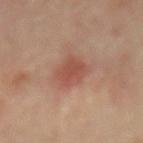Imaged during a routine full-body skin examination; the lesion was not biopsied and no histopathology is available. This image is a 15 mm lesion crop taken from a total-body photograph. The lesion-visualizer software estimated an average lesion color of about L≈49 a*≈24 b*≈27 (CIELAB) and a normalized lesion–skin contrast near 6.5. And it measured a nevus-likeness score of about 85/100 and a lesion-detection confidence of about 100/100. The lesion is located on the back. Approximately 3.5 mm at its widest. The tile uses cross-polarized illumination. A male patient, aged approximately 65.